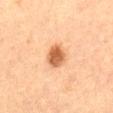The lesion was tiled from a total-body skin photograph and was not biopsied. A region of skin cropped from a whole-body photographic capture, roughly 15 mm wide. Captured under cross-polarized illumination. Located on the abdomen. A female patient aged 58 to 62. Measured at roughly 3 mm in maximum diameter.